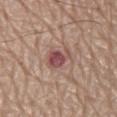| field | value |
|---|---|
| biopsy status | total-body-photography surveillance lesion; no biopsy |
| lesion size | about 3 mm |
| body site | the mid back |
| image source | total-body-photography crop, ~15 mm field of view |
| subject | male, roughly 80 years of age |
| illumination | white-light |